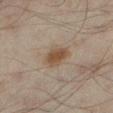Findings:
- notes · total-body-photography surveillance lesion; no biopsy
- location · the leg
- subject · male, in their mid- to late 40s
- image source · ~15 mm crop, total-body skin-cancer survey
- size · ~3.5 mm (longest diameter)
- TBP lesion metrics · a shape eccentricity near 0.8 and a shape-asymmetry score of about 0.25 (0 = symmetric); a nevus-likeness score of about 95/100 and lesion-presence confidence of about 100/100